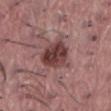No biopsy was performed on this lesion — it was imaged during a full skin examination and was not determined to be concerning. A male patient, in their 40s. Measured at roughly 4 mm in maximum diameter. This is a white-light tile. A 15 mm crop from a total-body photograph taken for skin-cancer surveillance. Located on the abdomen.